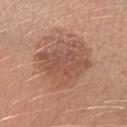  biopsy_status: not biopsied; imaged during a skin examination
  lighting: white-light
  automated_metrics:
    cielab_L: 53
    cielab_a: 21
    cielab_b: 29
    vs_skin_darker_L: 9.0
    vs_skin_contrast_norm: 6.0
    color_variation_0_10: 4.0
    peripheral_color_asymmetry: 1.0
  site: left forearm
  image:
    source: total-body photography crop
    field_of_view_mm: 15
  patient:
    sex: female
    age_approx: 25
  lesion_size:
    long_diameter_mm_approx: 7.0